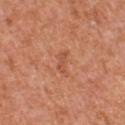Notes:
* biopsy status · no biopsy performed (imaged during a skin exam)
* site · the front of the torso
* automated lesion analysis · an automated nevus-likeness rating near 0 out of 100
* imaging modality · ~15 mm tile from a whole-body skin photo
* subject · male, in their mid- to late 50s
* tile lighting · white-light illumination
* lesion diameter · ~2.5 mm (longest diameter)